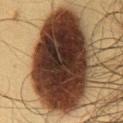Recorded during total-body skin imaging; not selected for excision or biopsy.
The lesion is on the chest.
The patient is a male aged approximately 35.
A close-up tile cropped from a whole-body skin photograph, about 15 mm across.